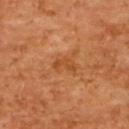follow-up = total-body-photography surveillance lesion; no biopsy | lesion size = ≈3 mm | site = the back | tile lighting = cross-polarized illumination | acquisition = ~15 mm tile from a whole-body skin photo | subject = female, in their mid-60s.